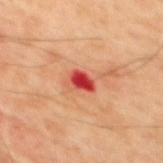Clinical impression:
Imaged during a routine full-body skin examination; the lesion was not biopsied and no histopathology is available.
Context:
Captured under cross-polarized illumination. On the mid back. A male patient, aged 43 to 47. Longest diameter approximately 2.5 mm. This image is a 15 mm lesion crop taken from a total-body photograph.